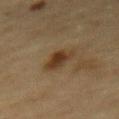Findings:
– patient · male, in their mid-80s or older
– anatomic site · the front of the torso
– image · ~15 mm crop, total-body skin-cancer survey